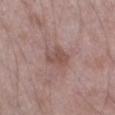  biopsy_status: not biopsied; imaged during a skin examination
  lighting: white-light
  lesion_size:
    long_diameter_mm_approx: 3.5
  site: leg
  patient:
    sex: male
    age_approx: 50
  image:
    source: total-body photography crop
    field_of_view_mm: 15